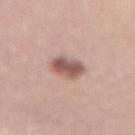Captured during whole-body skin photography for melanoma surveillance; the lesion was not biopsied. The lesion's longest dimension is about 3.5 mm. On the mid back. The patient is a male aged approximately 60. Automated image analysis of the tile measured an average lesion color of about L≈55 a*≈20 b*≈23 (CIELAB), about 14 CIELAB-L* units darker than the surrounding skin, and a normalized lesion–skin contrast near 9.5. The analysis additionally found a classifier nevus-likeness of about 85/100. A close-up tile cropped from a whole-body skin photograph, about 15 mm across. Captured under white-light illumination.